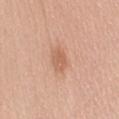| feature | finding |
|---|---|
| biopsy status | imaged on a skin check; not biopsied |
| patient | female, aged 63 to 67 |
| body site | the lower back |
| size | ~3 mm (longest diameter) |
| image | ~15 mm crop, total-body skin-cancer survey |
| tile lighting | white-light |